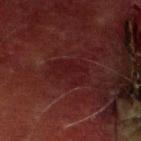Imaged during a routine full-body skin examination; the lesion was not biopsied and no histopathology is available. This image is a 15 mm lesion crop taken from a total-body photograph. This is a cross-polarized tile. The lesion's longest dimension is about 4 mm. The lesion-visualizer software estimated a lesion area of about 8 mm², an outline eccentricity of about 0.8 (0 = round, 1 = elongated), and two-axis asymmetry of about 0.3. The analysis additionally found a mean CIELAB color near L≈15 a*≈22 b*≈16 and a lesion–skin lightness drop of about 4. The analysis additionally found an automated nevus-likeness rating near 0 out of 100 and lesion-presence confidence of about 80/100. A male subject aged 68–72. From the right lower leg.